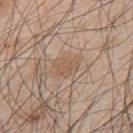notes: total-body-photography surveillance lesion; no biopsy | patient: male, aged 43 to 47 | automated metrics: an area of roughly 5 mm², an eccentricity of roughly 0.8, and two-axis asymmetry of about 0.25; a lesion color around L≈56 a*≈16 b*≈30 in CIELAB, a lesion–skin lightness drop of about 6, and a normalized lesion–skin contrast near 5.5; border irregularity of about 3.5 on a 0–10 scale and a color-variation rating of about 1.5/10; a lesion-detection confidence of about 90/100 | anatomic site: the back | imaging modality: 15 mm crop, total-body photography | illumination: white-light illumination | lesion diameter: ~3 mm (longest diameter).